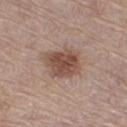The lesion was photographed on a routine skin check and not biopsied; there is no pathology result.
The tile uses white-light illumination.
Automated tile analysis of the lesion measured an area of roughly 13 mm², an eccentricity of roughly 0.55, and two-axis asymmetry of about 0.15. It also reported a border-irregularity index near 2/10, internal color variation of about 4 on a 0–10 scale, and radial color variation of about 1.5. The software also gave an automated nevus-likeness rating near 75 out of 100 and a lesion-detection confidence of about 100/100.
Located on the left leg.
Cropped from a total-body skin-imaging series; the visible field is about 15 mm.
A female subject approximately 65 years of age.
Measured at roughly 4.5 mm in maximum diameter.